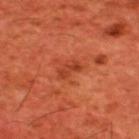Recorded during total-body skin imaging; not selected for excision or biopsy. The total-body-photography lesion software estimated a lesion area of about 4 mm², a shape eccentricity near 0.85, and two-axis asymmetry of about 0.4. And it measured a lesion color around L≈41 a*≈33 b*≈38 in CIELAB, roughly 8 lightness units darker than nearby skin, and a normalized lesion–skin contrast near 6. A lesion tile, about 15 mm wide, cut from a 3D total-body photograph. The lesion is located on the upper back. A male patient approximately 60 years of age. The tile uses cross-polarized illumination. The recorded lesion diameter is about 3 mm.